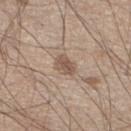Imaged during a routine full-body skin examination; the lesion was not biopsied and no histopathology is available. The tile uses white-light illumination. On the leg. A male subject, approximately 45 years of age. A 15 mm crop from a total-body photograph taken for skin-cancer surveillance.